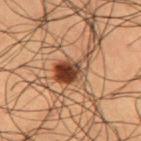{
  "biopsy_status": "not biopsied; imaged during a skin examination",
  "patient": {
    "sex": "male",
    "age_approx": 50
  },
  "automated_metrics": {
    "peripheral_color_asymmetry": 3.0
  },
  "image": {
    "source": "total-body photography crop",
    "field_of_view_mm": 15
  },
  "site": "left thigh",
  "lighting": "cross-polarized",
  "lesion_size": {
    "long_diameter_mm_approx": 5.0
  }
}